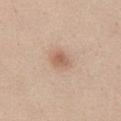Case summary:
- notes · total-body-photography surveillance lesion; no biopsy
- location · the chest
- image · total-body-photography crop, ~15 mm field of view
- subject · male, in their mid-30s